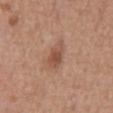This lesion was catalogued during total-body skin photography and was not selected for biopsy.
The lesion is located on the abdomen.
A male subject aged around 65.
Imaged with white-light lighting.
A 15 mm close-up extracted from a 3D total-body photography capture.
Automated image analysis of the tile measured an average lesion color of about L≈51 a*≈22 b*≈29 (CIELAB). And it measured a border-irregularity rating of about 2.5/10 and internal color variation of about 3 on a 0–10 scale. The software also gave a nevus-likeness score of about 75/100 and lesion-presence confidence of about 100/100.
The recorded lesion diameter is about 4 mm.